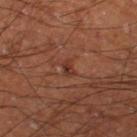The lesion was photographed on a routine skin check and not biopsied; there is no pathology result.
An algorithmic analysis of the crop reported a border-irregularity index near 2.5/10 and internal color variation of about 1 on a 0–10 scale.
About 2 mm across.
On the leg.
A region of skin cropped from a whole-body photographic capture, roughly 15 mm wide.
A male patient aged approximately 60.